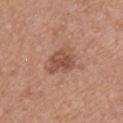Acquisition and patient details: Imaged with white-light lighting. A lesion tile, about 15 mm wide, cut from a 3D total-body photograph. The lesion's longest dimension is about 3.5 mm. A female subject in their mid-30s. The lesion is on the upper back.